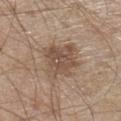<case>
<biopsy_status>not biopsied; imaged during a skin examination</biopsy_status>
<image>
  <source>total-body photography crop</source>
  <field_of_view_mm>15</field_of_view_mm>
</image>
<patient>
  <sex>male</sex>
  <age_approx>60</age_approx>
</patient>
<site>left lower leg</site>
</case>